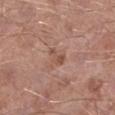biopsy status: catalogued during a skin exam; not biopsied | body site: the left lower leg | lesion size: ≈2.5 mm | image-analysis metrics: an average lesion color of about L≈51 a*≈21 b*≈27 (CIELAB) and a normalized lesion–skin contrast near 6 | image source: ~15 mm crop, total-body skin-cancer survey | patient: male, roughly 55 years of age | lighting: white-light.